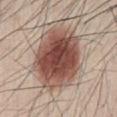Part of a total-body skin-imaging series; this lesion was reviewed on a skin check and was not flagged for biopsy.
On the abdomen.
A lesion tile, about 15 mm wide, cut from a 3D total-body photograph.
A male subject about 25 years old.